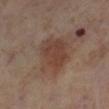Notes:
- follow-up · imaged on a skin check; not biopsied
- image source · 15 mm crop, total-body photography
- subject · female, aged around 55
- site · the right lower leg